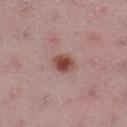subject: female, aged around 45 | image source: 15 mm crop, total-body photography | site: the left lower leg.